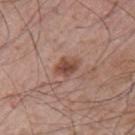Clinical impression: Imaged during a routine full-body skin examination; the lesion was not biopsied and no histopathology is available. Image and clinical context: Measured at roughly 3 mm in maximum diameter. The tile uses white-light illumination. A male patient aged 63–67. This image is a 15 mm lesion crop taken from a total-body photograph. From the arm. Automated image analysis of the tile measured a lesion color around L≈47 a*≈22 b*≈27 in CIELAB, a lesion–skin lightness drop of about 11, and a normalized border contrast of about 8.5.